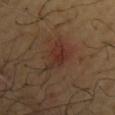This lesion was catalogued during total-body skin photography and was not selected for biopsy.
A male subject in their mid- to late 60s.
A 15 mm close-up extracted from a 3D total-body photography capture.
The recorded lesion diameter is about 5 mm.
From the back.
Captured under cross-polarized illumination.